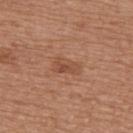notes: catalogued during a skin exam; not biopsied | site: the upper back | TBP lesion metrics: a border-irregularity index near 3.5/10, internal color variation of about 3 on a 0–10 scale, and radial color variation of about 1 | patient: male, roughly 65 years of age | tile lighting: white-light illumination | lesion size: ~3.5 mm (longest diameter) | image source: ~15 mm crop, total-body skin-cancer survey.